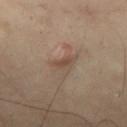The lesion was photographed on a routine skin check and not biopsied; there is no pathology result.
Automated tile analysis of the lesion measured a lesion color around L≈46 a*≈16 b*≈26 in CIELAB and a normalized border contrast of about 6. The analysis additionally found peripheral color asymmetry of about 0.5. And it measured a lesion-detection confidence of about 100/100.
A close-up tile cropped from a whole-body skin photograph, about 15 mm across.
About 2.5 mm across.
A male subject aged 48 to 52.
From the upper back.
The tile uses cross-polarized illumination.